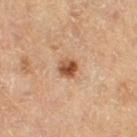No biopsy was performed on this lesion — it was imaged during a full skin examination and was not determined to be concerning.
The lesion is on the right thigh.
The patient is a female aged around 65.
Approximately 2.5 mm at its widest.
The tile uses cross-polarized illumination.
This image is a 15 mm lesion crop taken from a total-body photograph.
Automated tile analysis of the lesion measured a footprint of about 4.5 mm² and a symmetry-axis asymmetry near 0.25. The analysis additionally found a within-lesion color-variation index near 8/10. It also reported lesion-presence confidence of about 100/100.